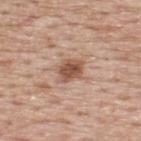A lesion tile, about 15 mm wide, cut from a 3D total-body photograph. Approximately 3 mm at its widest. Located on the upper back. A male subject roughly 75 years of age.